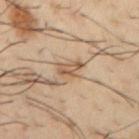imaging modality — 15 mm crop, total-body photography; subject — male, aged approximately 40; illumination — cross-polarized; lesion diameter — about 3 mm; site — the arm.